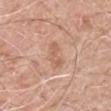Part of a total-body skin-imaging series; this lesion was reviewed on a skin check and was not flagged for biopsy. A close-up tile cropped from a whole-body skin photograph, about 15 mm across. The patient is a male about 60 years old. Located on the right upper arm.